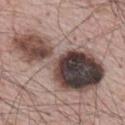Recorded during total-body skin imaging; not selected for excision or biopsy. An algorithmic analysis of the crop reported a border-irregularity rating of about 7/10, a color-variation rating of about 10/10, and peripheral color asymmetry of about 4.5. It also reported an automated nevus-likeness rating near 10 out of 100 and a lesion-detection confidence of about 95/100. On the upper back. Measured at roughly 12.5 mm in maximum diameter. A lesion tile, about 15 mm wide, cut from a 3D total-body photograph. A male subject in their mid-60s. This is a white-light tile.